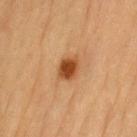Impression: Part of a total-body skin-imaging series; this lesion was reviewed on a skin check and was not flagged for biopsy. Context: A male subject roughly 85 years of age or older. The lesion is located on the left upper arm. Longest diameter approximately 3 mm. A close-up tile cropped from a whole-body skin photograph, about 15 mm across. Imaged with cross-polarized lighting.